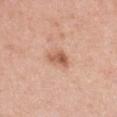notes = imaged on a skin check; not biopsied | subject = female, in their 40s | tile lighting = white-light illumination | site = the chest | automated metrics = a footprint of about 4 mm², a shape eccentricity near 0.7, and a symmetry-axis asymmetry near 0.35; a lesion color around L≈58 a*≈24 b*≈33 in CIELAB, about 12 CIELAB-L* units darker than the surrounding skin, and a normalized border contrast of about 8; a border-irregularity rating of about 3.5/10, internal color variation of about 5 on a 0–10 scale, and peripheral color asymmetry of about 2 | image source = total-body-photography crop, ~15 mm field of view | diameter = ~2.5 mm (longest diameter).